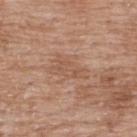Notes:
– biopsy status · catalogued during a skin exam; not biopsied
– tile lighting · white-light illumination
– acquisition · ~15 mm tile from a whole-body skin photo
– image-analysis metrics · a lesion area of about 9.5 mm² and a symmetry-axis asymmetry near 0.4; a border-irregularity rating of about 4.5/10 and internal color variation of about 2.5 on a 0–10 scale; a nevus-likeness score of about 0/100 and lesion-presence confidence of about 100/100
– location · the upper back
– subject · male, aged around 50
– lesion diameter · about 5 mm